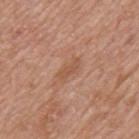imaging modality = 15 mm crop, total-body photography
size = ≈3 mm
TBP lesion metrics = a lesion-detection confidence of about 100/100
body site = the mid back
patient = male, in their 60s